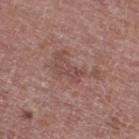notes: catalogued during a skin exam; not biopsied
lesion diameter: about 6 mm
image source: 15 mm crop, total-body photography
automated lesion analysis: an area of roughly 10 mm², an eccentricity of roughly 0.9, and two-axis asymmetry of about 0.6; a border-irregularity index near 8/10, a within-lesion color-variation index near 2.5/10, and radial color variation of about 1
body site: the leg
patient: male, aged 73 to 77